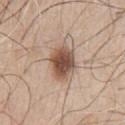notes = total-body-photography surveillance lesion; no biopsy | acquisition = 15 mm crop, total-body photography | patient = male, in their mid-60s | location = the chest | lesion diameter = ~4.5 mm (longest diameter).